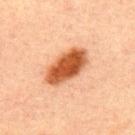Imaged during a routine full-body skin examination; the lesion was not biopsied and no histopathology is available. The lesion is on the upper back. The patient is a male aged 28–32. A 15 mm close-up extracted from a 3D total-body photography capture.